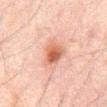• workup — imaged on a skin check; not biopsied
• automated lesion analysis — a lesion area of about 6.5 mm², a shape eccentricity near 0.55, and a symmetry-axis asymmetry near 0.25; a border-irregularity index near 2/10, internal color variation of about 5 on a 0–10 scale, and peripheral color asymmetry of about 1.5
• image source — ~15 mm crop, total-body skin-cancer survey
• anatomic site — the abdomen
• tile lighting — cross-polarized
• subject — male, aged approximately 60